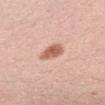The lesion-visualizer software estimated an area of roughly 6 mm², an eccentricity of roughly 0.65, and a symmetry-axis asymmetry near 0.2. And it measured a border-irregularity index near 2/10, a color-variation rating of about 3.5/10, and peripheral color asymmetry of about 1. The analysis additionally found a classifier nevus-likeness of about 95/100 and a detector confidence of about 100 out of 100 that the crop contains a lesion. Cropped from a whole-body photographic skin survey; the tile spans about 15 mm. On the right upper arm. About 3 mm across. A female subject aged around 35.